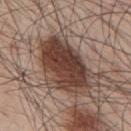Impression: The lesion was tiled from a total-body skin photograph and was not biopsied. Acquisition and patient details: A male patient approximately 55 years of age. On the upper back. The lesion-visualizer software estimated a footprint of about 27 mm², a shape eccentricity near 0.8, and two-axis asymmetry of about 0.15. It also reported a border-irregularity index near 2.5/10, a within-lesion color-variation index near 5.5/10, and a peripheral color-asymmetry measure near 2. The software also gave a classifier nevus-likeness of about 70/100 and lesion-presence confidence of about 100/100. A roughly 15 mm field-of-view crop from a total-body skin photograph. Imaged with white-light lighting. The recorded lesion diameter is about 7.5 mm.